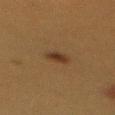Assessment:
No biopsy was performed on this lesion — it was imaged during a full skin examination and was not determined to be concerning.
Image and clinical context:
Imaged with cross-polarized lighting. The subject is a female aged 38 to 42. The lesion is on the mid back. Automated tile analysis of the lesion measured a footprint of about 4 mm², an eccentricity of roughly 0.7, and a symmetry-axis asymmetry near 0.2. The software also gave an average lesion color of about L≈28 a*≈15 b*≈26 (CIELAB) and about 8 CIELAB-L* units darker than the surrounding skin. The software also gave a border-irregularity rating of about 2/10, a color-variation rating of about 2/10, and a peripheral color-asymmetry measure near 0.5. The software also gave a lesion-detection confidence of about 100/100. A 15 mm close-up extracted from a 3D total-body photography capture.